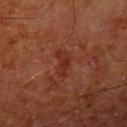The lesion was tiled from a total-body skin photograph and was not biopsied. A male patient aged 78–82. A roughly 15 mm field-of-view crop from a total-body skin photograph. The lesion is on the left upper arm. Automated tile analysis of the lesion measured an outline eccentricity of about 0.9 (0 = round, 1 = elongated) and a shape-asymmetry score of about 0.35 (0 = symmetric). The software also gave a lesion color around L≈23 a*≈22 b*≈25 in CIELAB and a normalized border contrast of about 6. The analysis additionally found a border-irregularity rating of about 4.5/10, a within-lesion color-variation index near 2/10, and peripheral color asymmetry of about 0.5. The software also gave an automated nevus-likeness rating near 0 out of 100 and lesion-presence confidence of about 100/100. Imaged with cross-polarized lighting.